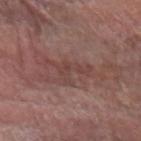biopsy status: no biopsy performed (imaged during a skin exam); lesion diameter: about 5 mm; site: the arm; patient: male, about 80 years old; imaging modality: total-body-photography crop, ~15 mm field of view; lighting: white-light illumination.